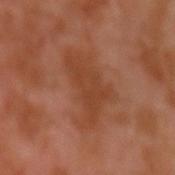The lesion was photographed on a routine skin check and not biopsied; there is no pathology result.
The lesion is located on the left upper arm.
Cropped from a whole-body photographic skin survey; the tile spans about 15 mm.
The patient is a male aged around 30.
Captured under cross-polarized illumination.
Approximately 7 mm at its widest.